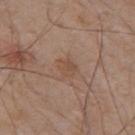Captured during whole-body skin photography for melanoma surveillance; the lesion was not biopsied. A lesion tile, about 15 mm wide, cut from a 3D total-body photograph. On the back. The patient is a male aged approximately 70.